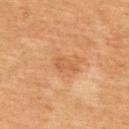Clinical impression:
The lesion was tiled from a total-body skin photograph and was not biopsied.
Background:
Cropped from a whole-body photographic skin survey; the tile spans about 15 mm. This is a cross-polarized tile. The patient is a male aged 58–62. The total-body-photography lesion software estimated a footprint of about 3 mm² and a shape-asymmetry score of about 0.4 (0 = symmetric). The software also gave about 7 CIELAB-L* units darker than the surrounding skin and a normalized lesion–skin contrast near 5. The analysis additionally found an automated nevus-likeness rating near 0 out of 100 and a detector confidence of about 100 out of 100 that the crop contains a lesion. The recorded lesion diameter is about 2.5 mm. From the back.